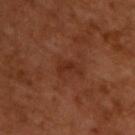| field | value |
|---|---|
| follow-up | no biopsy performed (imaged during a skin exam) |
| subject | male, approximately 50 years of age |
| lesion size | ~3 mm (longest diameter) |
| location | the back |
| image | 15 mm crop, total-body photography |
| tile lighting | cross-polarized |